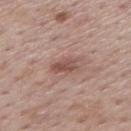location: the mid back; illumination: white-light illumination; size: about 3.5 mm; image source: ~15 mm tile from a whole-body skin photo; patient: male, in their mid-70s.